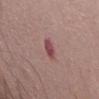{"biopsy_status": "not biopsied; imaged during a skin examination", "lesion_size": {"long_diameter_mm_approx": 3.0}, "patient": {"sex": "female", "age_approx": 50}, "site": "abdomen", "lighting": "white-light", "image": {"source": "total-body photography crop", "field_of_view_mm": 15}}